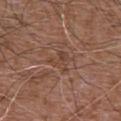Impression:
This lesion was catalogued during total-body skin photography and was not selected for biopsy.
Image and clinical context:
A male subject approximately 75 years of age. Automated tile analysis of the lesion measured a normalized lesion–skin contrast near 5. The analysis additionally found a border-irregularity rating of about 5.5/10, internal color variation of about 2.5 on a 0–10 scale, and a peripheral color-asymmetry measure near 1. Cropped from a whole-body photographic skin survey; the tile spans about 15 mm. On the abdomen.